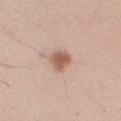notes = catalogued during a skin exam; not biopsied
patient = male, in their mid- to late 40s
diameter = about 3 mm
lighting = white-light
acquisition = 15 mm crop, total-body photography
anatomic site = the right upper arm